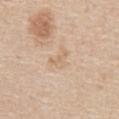biopsy status: no biopsy performed (imaged during a skin exam) | subject: male, aged 63 to 67 | site: the abdomen | image source: ~15 mm tile from a whole-body skin photo | size: about 3 mm.